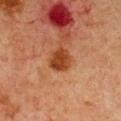Notes:
* notes: catalogued during a skin exam; not biopsied
* lighting: cross-polarized illumination
* imaging modality: ~15 mm tile from a whole-body skin photo
* patient: female, aged approximately 40
* diameter: about 3 mm
* location: the chest